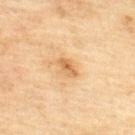workup: total-body-photography surveillance lesion; no biopsy | lesion size: ≈2.5 mm | image: ~15 mm tile from a whole-body skin photo | patient: male, roughly 45 years of age | location: the upper back | lighting: cross-polarized illumination.